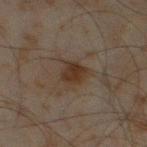<record>
  <biopsy_status>not biopsied; imaged during a skin examination</biopsy_status>
  <site>leg</site>
  <image>
    <source>total-body photography crop</source>
    <field_of_view_mm>15</field_of_view_mm>
  </image>
  <patient>
    <sex>male</sex>
    <age_approx>45</age_approx>
  </patient>
</record>